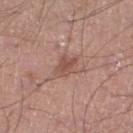  biopsy_status: not biopsied; imaged during a skin examination
  patient:
    sex: male
    age_approx: 55
  site: right lower leg
  lighting: white-light
  image:
    source: total-body photography crop
    field_of_view_mm: 15
  lesion_size:
    long_diameter_mm_approx: 3.0